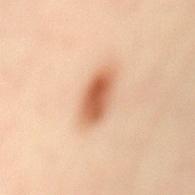subject: female, aged around 40 | lesion diameter: about 5 mm | image-analysis metrics: a symmetry-axis asymmetry near 0.15; a mean CIELAB color near L≈61 a*≈25 b*≈36 and a lesion-to-skin contrast of about 10 (normalized; higher = more distinct) | illumination: cross-polarized illumination | image source: ~15 mm tile from a whole-body skin photo | site: the mid back.